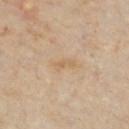| feature | finding |
|---|---|
| notes | no biopsy performed (imaged during a skin exam) |